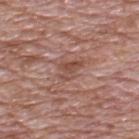No biopsy was performed on this lesion — it was imaged during a full skin examination and was not determined to be concerning. Longest diameter approximately 3.5 mm. Automated tile analysis of the lesion measured a lesion-to-skin contrast of about 6 (normalized; higher = more distinct). The software also gave a border-irregularity index near 5.5/10, internal color variation of about 4 on a 0–10 scale, and a peripheral color-asymmetry measure near 1.5. The subject is a male about 70 years old. The tile uses white-light illumination. A 15 mm close-up extracted from a 3D total-body photography capture. The lesion is on the upper back.